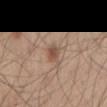Assessment:
Part of a total-body skin-imaging series; this lesion was reviewed on a skin check and was not flagged for biopsy.
Context:
The lesion is located on the right thigh. This image is a 15 mm lesion crop taken from a total-body photograph. A male patient aged around 60. This is a white-light tile.